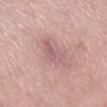Notes:
– follow-up — total-body-photography surveillance lesion; no biopsy